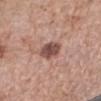Recorded during total-body skin imaging; not selected for excision or biopsy. Imaged with white-light lighting. The lesion's longest dimension is about 3 mm. The lesion is located on the right forearm. The patient is a female aged 58 to 62. A roughly 15 mm field-of-view crop from a total-body skin photograph.